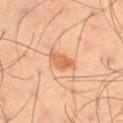The lesion was photographed on a routine skin check and not biopsied; there is no pathology result. A region of skin cropped from a whole-body photographic capture, roughly 15 mm wide. The subject is a male in their mid- to late 50s. The lesion is on the left thigh.